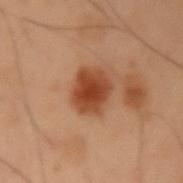No biopsy was performed on this lesion — it was imaged during a full skin examination and was not determined to be concerning. Automated image analysis of the tile measured a lesion color around L≈35 a*≈22 b*≈29 in CIELAB, about 11 CIELAB-L* units darker than the surrounding skin, and a normalized lesion–skin contrast near 10.5. And it measured border irregularity of about 2 on a 0–10 scale, a within-lesion color-variation index near 3/10, and a peripheral color-asymmetry measure near 0.5. The analysis additionally found an automated nevus-likeness rating near 95 out of 100 and a lesion-detection confidence of about 100/100. A male patient in their mid- to late 50s. Approximately 4 mm at its widest. The lesion is located on the left upper arm. Cropped from a total-body skin-imaging series; the visible field is about 15 mm. Imaged with cross-polarized lighting.